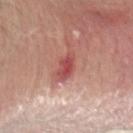follow-up = no biopsy performed (imaged during a skin exam)
acquisition = total-body-photography crop, ~15 mm field of view
size = ~3 mm (longest diameter)
illumination = white-light illumination
body site = the head or neck
subject = male, approximately 65 years of age
automated metrics = an average lesion color of about L≈50 a*≈32 b*≈25 (CIELAB) and a normalized border contrast of about 8